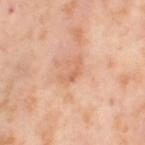{
  "automated_metrics": {
    "cielab_L": 65,
    "cielab_a": 24,
    "cielab_b": 34,
    "vs_skin_darker_L": 8.0,
    "vs_skin_contrast_norm": 5.0,
    "border_irregularity_0_10": 7.0,
    "peripheral_color_asymmetry": 0.0,
    "nevus_likeness_0_100": 0,
    "lesion_detection_confidence_0_100": 100
  },
  "lesion_size": {
    "long_diameter_mm_approx": 3.0
  },
  "site": "left thigh",
  "patient": {
    "sex": "female",
    "age_approx": 55
  },
  "image": {
    "source": "total-body photography crop",
    "field_of_view_mm": 15
  }
}